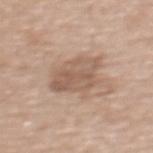Impression: This lesion was catalogued during total-body skin photography and was not selected for biopsy.